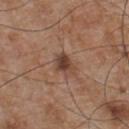The lesion was photographed on a routine skin check and not biopsied; there is no pathology result. Cropped from a whole-body photographic skin survey; the tile spans about 15 mm. Automated tile analysis of the lesion measured a footprint of about 5.5 mm², a shape eccentricity near 0.7, and a symmetry-axis asymmetry near 0.3. The analysis additionally found a lesion–skin lightness drop of about 11 and a normalized border contrast of about 8.5. Measured at roughly 3 mm in maximum diameter. Imaged with white-light lighting. The lesion is located on the chest. The subject is a male approximately 55 years of age.